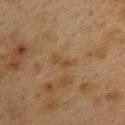The recorded lesion diameter is about 3 mm. A 15 mm close-up tile from a total-body photography series done for melanoma screening. A female subject, about 40 years old. Automated image analysis of the tile measured a within-lesion color-variation index near 0/10 and a peripheral color-asymmetry measure near 0. Located on the upper back.